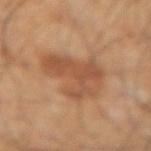workup — imaged on a skin check; not biopsied | tile lighting — cross-polarized | lesion size — ~7 mm (longest diameter) | subject — male, aged around 55 | image source — ~15 mm crop, total-body skin-cancer survey | body site — the left forearm.